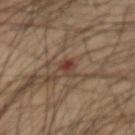biopsy status: catalogued during a skin exam; not biopsied | subject: male, roughly 60 years of age | lighting: cross-polarized illumination | TBP lesion metrics: an average lesion color of about L≈38 a*≈19 b*≈25 (CIELAB), a lesion–skin lightness drop of about 8, and a normalized lesion–skin contrast near 7 | size: ≈2.5 mm | image: ~15 mm tile from a whole-body skin photo | location: the abdomen.